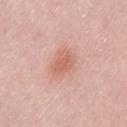biopsy status: total-body-photography surveillance lesion; no biopsy.